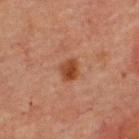Part of a total-body skin-imaging series; this lesion was reviewed on a skin check and was not flagged for biopsy.
Located on the upper back.
A male patient, about 60 years old.
A 15 mm close-up extracted from a 3D total-body photography capture.
This is a cross-polarized tile.